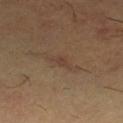Recorded during total-body skin imaging; not selected for excision or biopsy.
A lesion tile, about 15 mm wide, cut from a 3D total-body photograph.
Captured under cross-polarized illumination.
Longest diameter approximately 3 mm.
A male patient aged approximately 60.
Located on the left thigh.